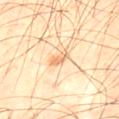notes: catalogued during a skin exam; not biopsied
subject: male, approximately 55 years of age
lesion diameter: ~3 mm (longest diameter)
illumination: cross-polarized
anatomic site: the left thigh
imaging modality: ~15 mm crop, total-body skin-cancer survey